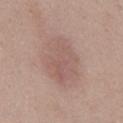biopsy_status: not biopsied; imaged during a skin examination
site: front of the torso
lighting: white-light
image:
  source: total-body photography crop
  field_of_view_mm: 15
patient:
  sex: female
  age_approx: 40
lesion_size:
  long_diameter_mm_approx: 6.0